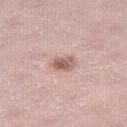* notes: no biopsy performed (imaged during a skin exam)
* body site: the right thigh
* subject: female, aged 38 to 42
* lighting: white-light
* acquisition: ~15 mm crop, total-body skin-cancer survey
* automated metrics: a mean CIELAB color near L≈60 a*≈20 b*≈24, a lesion–skin lightness drop of about 12, and a lesion-to-skin contrast of about 8 (normalized; higher = more distinct); border irregularity of about 2 on a 0–10 scale, internal color variation of about 5 on a 0–10 scale, and peripheral color asymmetry of about 1.5; an automated nevus-likeness rating near 90 out of 100